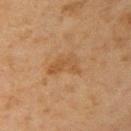lighting: cross-polarized
automated metrics: a mean CIELAB color near L≈46 a*≈17 b*≈34, a lesion–skin lightness drop of about 6, and a normalized border contrast of about 5.5; a classifier nevus-likeness of about 0/100 and a detector confidence of about 100 out of 100 that the crop contains a lesion
image source: ~15 mm crop, total-body skin-cancer survey
site: the right upper arm
subject: female, roughly 55 years of age